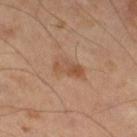Clinical impression: This lesion was catalogued during total-body skin photography and was not selected for biopsy. Acquisition and patient details: This image is a 15 mm lesion crop taken from a total-body photograph. Located on the right thigh. A male subject, aged 63–67. Approximately 4 mm at its widest.